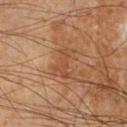Assessment:
Recorded during total-body skin imaging; not selected for excision or biopsy.
Clinical summary:
Located on the right lower leg. Captured under cross-polarized illumination. A male patient about 60 years old. A 15 mm close-up tile from a total-body photography series done for melanoma screening.